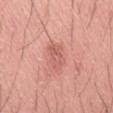No biopsy was performed on this lesion — it was imaged during a full skin examination and was not determined to be concerning.
From the abdomen.
Cropped from a total-body skin-imaging series; the visible field is about 15 mm.
The lesion's longest dimension is about 4 mm.
Captured under white-light illumination.
The lesion-visualizer software estimated a lesion color around L≈60 a*≈29 b*≈27 in CIELAB, a lesion–skin lightness drop of about 9, and a lesion-to-skin contrast of about 5.5 (normalized; higher = more distinct). The software also gave a border-irregularity rating of about 3.5/10, internal color variation of about 4.5 on a 0–10 scale, and radial color variation of about 2. The analysis additionally found a nevus-likeness score of about 5/100 and a detector confidence of about 100 out of 100 that the crop contains a lesion.
A male patient roughly 45 years of age.